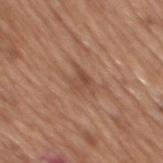<record>
<biopsy_status>not biopsied; imaged during a skin examination</biopsy_status>
</record>